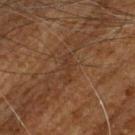notes=catalogued during a skin exam; not biopsied
image source=15 mm crop, total-body photography
body site=the head or neck
patient=male, aged around 65